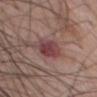{
  "biopsy_status": "not biopsied; imaged during a skin examination",
  "site": "chest",
  "image": {
    "source": "total-body photography crop",
    "field_of_view_mm": 15
  },
  "patient": {
    "sex": "male",
    "age_approx": 60
  }
}